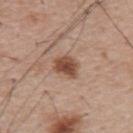Q: Was a biopsy performed?
A: no biopsy performed (imaged during a skin exam)
Q: How large is the lesion?
A: ≈3.5 mm
Q: What kind of image is this?
A: 15 mm crop, total-body photography
Q: What did automated image analysis measure?
A: a mean CIELAB color near L≈48 a*≈21 b*≈29, a lesion–skin lightness drop of about 14, and a lesion-to-skin contrast of about 10 (normalized; higher = more distinct); internal color variation of about 3.5 on a 0–10 scale and a peripheral color-asymmetry measure near 1; an automated nevus-likeness rating near 95 out of 100 and a detector confidence of about 100 out of 100 that the crop contains a lesion
Q: Who is the patient?
A: male, aged approximately 55
Q: What lighting was used for the tile?
A: white-light illumination